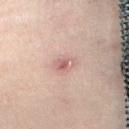{
  "biopsy_status": "not biopsied; imaged during a skin examination",
  "patient": {
    "sex": "female",
    "age_approx": 40
  },
  "lesion_size": {
    "long_diameter_mm_approx": 2.5
  },
  "lighting": "cross-polarized",
  "site": "abdomen",
  "image": {
    "source": "total-body photography crop",
    "field_of_view_mm": 15
  }
}